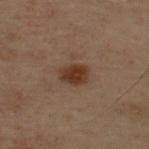| field | value |
|---|---|
| TBP lesion metrics | a shape eccentricity near 0.65 and a shape-asymmetry score of about 0.2 (0 = symmetric); an average lesion color of about L≈28 a*≈16 b*≈24 (CIELAB), a lesion–skin lightness drop of about 9, and a lesion-to-skin contrast of about 10 (normalized; higher = more distinct) |
| illumination | cross-polarized |
| acquisition | ~15 mm tile from a whole-body skin photo |
| patient | male, approximately 50 years of age |
| size | about 3 mm |
| anatomic site | the upper back |